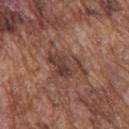• biopsy status — total-body-photography surveillance lesion; no biopsy
• lesion size — ~5.5 mm (longest diameter)
• lighting — white-light illumination
• TBP lesion metrics — a lesion area of about 12 mm², an outline eccentricity of about 0.7 (0 = round, 1 = elongated), and two-axis asymmetry of about 0.5; a classifier nevus-likeness of about 15/100 and a lesion-detection confidence of about 90/100
• patient — male, roughly 75 years of age
• imaging modality — total-body-photography crop, ~15 mm field of view
• site — the upper back